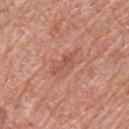Clinical summary: A lesion tile, about 15 mm wide, cut from a 3D total-body photograph. On the upper back. The total-body-photography lesion software estimated an average lesion color of about L≈53 a*≈26 b*≈30 (CIELAB), roughly 8 lightness units darker than nearby skin, and a normalized lesion–skin contrast near 5.5. It also reported a border-irregularity rating of about 5/10, a color-variation rating of about 2.5/10, and peripheral color asymmetry of about 1. The analysis additionally found an automated nevus-likeness rating near 0 out of 100 and a detector confidence of about 100 out of 100 that the crop contains a lesion. The subject is a male approximately 60 years of age.